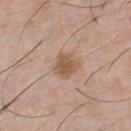The lesion is located on the chest.
A male patient, in their mid- to late 40s.
This is a white-light tile.
A close-up tile cropped from a whole-body skin photograph, about 15 mm across.